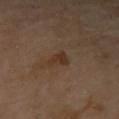Impression:
The lesion was tiled from a total-body skin photograph and was not biopsied.
Background:
An algorithmic analysis of the crop reported a lesion area of about 3 mm², an outline eccentricity of about 0.8 (0 = round, 1 = elongated), and a shape-asymmetry score of about 0.5 (0 = symmetric). And it measured a lesion color around L≈33 a*≈17 b*≈27 in CIELAB and a lesion–skin lightness drop of about 7. And it measured a classifier nevus-likeness of about 30/100 and a lesion-detection confidence of about 100/100. The tile uses cross-polarized illumination. The lesion is on the right forearm. A female patient, aged 68 to 72. A 15 mm close-up extracted from a 3D total-body photography capture.